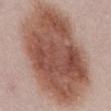Q: Was this lesion biopsied?
A: catalogued during a skin exam; not biopsied
Q: Lesion location?
A: the front of the torso
Q: What are the patient's age and sex?
A: female, aged approximately 50
Q: What kind of image is this?
A: ~15 mm tile from a whole-body skin photo